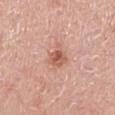Notes:
– workup · no biopsy performed (imaged during a skin exam)
– patient · male, aged approximately 70
– anatomic site · the left lower leg
– TBP lesion metrics · a mean CIELAB color near L≈58 a*≈25 b*≈29, roughly 11 lightness units darker than nearby skin, and a normalized border contrast of about 7
– acquisition · total-body-photography crop, ~15 mm field of view